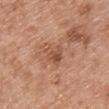<lesion>
<biopsy_status>not biopsied; imaged during a skin examination</biopsy_status>
</lesion>